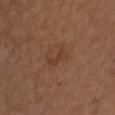The lesion was tiled from a total-body skin photograph and was not biopsied.
A lesion tile, about 15 mm wide, cut from a 3D total-body photograph.
About 3 mm across.
The subject is a male in their mid-70s.
Imaged with white-light lighting.
On the right upper arm.